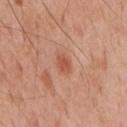Imaged during a routine full-body skin examination; the lesion was not biopsied and no histopathology is available.
Measured at roughly 2.5 mm in maximum diameter.
A male subject, approximately 55 years of age.
A 15 mm close-up tile from a total-body photography series done for melanoma screening.
Automated image analysis of the tile measured an area of roughly 3.5 mm² and a shape-asymmetry score of about 0.25 (0 = symmetric). The software also gave a lesion color around L≈54 a*≈27 b*≈32 in CIELAB, a lesion–skin lightness drop of about 9, and a lesion-to-skin contrast of about 6.5 (normalized; higher = more distinct).
The lesion is located on the chest.